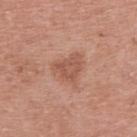The lesion was photographed on a routine skin check and not biopsied; there is no pathology result. The tile uses white-light illumination. Located on the upper back. The lesion's longest dimension is about 4 mm. A female subject, aged 68 to 72. This image is a 15 mm lesion crop taken from a total-body photograph. The lesion-visualizer software estimated a footprint of about 8.5 mm² and two-axis asymmetry of about 0.4. The software also gave a lesion color around L≈54 a*≈24 b*≈29 in CIELAB and a normalized border contrast of about 6. The software also gave a border-irregularity rating of about 4/10, a color-variation rating of about 2.5/10, and a peripheral color-asymmetry measure near 1.